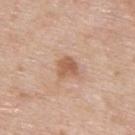site: the upper back | image: ~15 mm crop, total-body skin-cancer survey | subject: male, aged approximately 60.